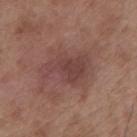{"biopsy_status": "not biopsied; imaged during a skin examination", "image": {"source": "total-body photography crop", "field_of_view_mm": 15}, "lighting": "white-light", "patient": {"sex": "male", "age_approx": 55}, "site": "mid back"}